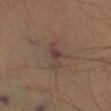Q: Was this lesion biopsied?
A: catalogued during a skin exam; not biopsied
Q: Automated lesion metrics?
A: a footprint of about 4.5 mm² and a symmetry-axis asymmetry near 0.65; a mean CIELAB color near L≈37 a*≈14 b*≈20, a lesion–skin lightness drop of about 6, and a normalized border contrast of about 5.5; a classifier nevus-likeness of about 0/100 and a detector confidence of about 100 out of 100 that the crop contains a lesion
Q: Patient demographics?
A: male, about 45 years old
Q: Where on the body is the lesion?
A: the right lower leg
Q: What is the imaging modality?
A: total-body-photography crop, ~15 mm field of view